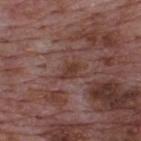<record>
<biopsy_status>not biopsied; imaged during a skin examination</biopsy_status>
<lighting>white-light</lighting>
<lesion_size>
  <long_diameter_mm_approx>3.0</long_diameter_mm_approx>
</lesion_size>
<image>
  <source>total-body photography crop</source>
  <field_of_view_mm>15</field_of_view_mm>
</image>
<patient>
  <sex>male</sex>
  <age_approx>70</age_approx>
</patient>
<site>upper back</site>
</record>